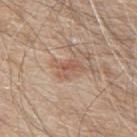notes = no biopsy performed (imaged during a skin exam); subject = male, aged approximately 80; illumination = white-light illumination; lesion size = about 4 mm; acquisition = ~15 mm tile from a whole-body skin photo; location = the upper back.